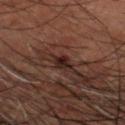Imaged during a routine full-body skin examination; the lesion was not biopsied and no histopathology is available.
On the head or neck.
Cropped from a whole-body photographic skin survey; the tile spans about 15 mm.
A male patient, aged 58–62.
The lesion's longest dimension is about 2.5 mm.